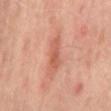Case summary:
– notes — catalogued during a skin exam; not biopsied
– image — ~15 mm crop, total-body skin-cancer survey
– site — the mid back
– patient — male, roughly 65 years of age